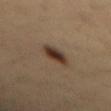follow-up: total-body-photography surveillance lesion; no biopsy | TBP lesion metrics: a footprint of about 6.5 mm² and a symmetry-axis asymmetry near 0.15; a lesion color around L≈32 a*≈14 b*≈23 in CIELAB, about 11 CIELAB-L* units darker than the surrounding skin, and a normalized border contrast of about 10.5; a nevus-likeness score of about 100/100 and lesion-presence confidence of about 100/100 | site: the mid back | lighting: cross-polarized | imaging modality: total-body-photography crop, ~15 mm field of view | size: ≈3.5 mm | patient: male, aged 33 to 37.